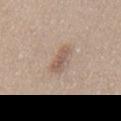Imaged during a routine full-body skin examination; the lesion was not biopsied and no histopathology is available.
The total-body-photography lesion software estimated a lesion area of about 4.5 mm², a shape eccentricity near 0.95, and a symmetry-axis asymmetry near 0.3. The analysis additionally found an average lesion color of about L≈56 a*≈16 b*≈26 (CIELAB) and roughly 10 lightness units darker than nearby skin. The software also gave a color-variation rating of about 1.5/10 and a peripheral color-asymmetry measure near 0.
Imaged with white-light lighting.
The subject is a male aged 43–47.
A region of skin cropped from a whole-body photographic capture, roughly 15 mm wide.
The lesion is located on the chest.